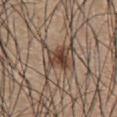biopsy status=imaged on a skin check; not biopsied | site=the front of the torso | patient=male, roughly 20 years of age | image=~15 mm tile from a whole-body skin photo | lighting=white-light illumination | TBP lesion metrics=an eccentricity of roughly 0.7 and two-axis asymmetry of about 0.25; a lesion–skin lightness drop of about 11 and a normalized border contrast of about 8.5 | lesion size=about 4.5 mm.